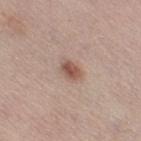biopsy_status: not biopsied; imaged during a skin examination
image:
  source: total-body photography crop
  field_of_view_mm: 15
site: right thigh
lighting: white-light
patient:
  sex: female
  age_approx: 30